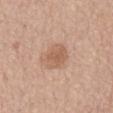Imaged during a routine full-body skin examination; the lesion was not biopsied and no histopathology is available. A 15 mm close-up tile from a total-body photography series done for melanoma screening. The recorded lesion diameter is about 3.5 mm. The subject is a male aged around 65. The lesion is on the back.